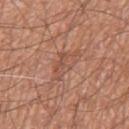<lesion>
  <biopsy_status>not biopsied; imaged during a skin examination</biopsy_status>
  <site>right thigh</site>
  <patient>
    <sex>male</sex>
    <age_approx>55</age_approx>
  </patient>
  <image>
    <source>total-body photography crop</source>
    <field_of_view_mm>15</field_of_view_mm>
  </image>
  <lighting>white-light</lighting>
  <lesion_size>
    <long_diameter_mm_approx>4.5</long_diameter_mm_approx>
  </lesion_size>
  <automated_metrics>
    <border_irregularity_0_10>4.5</border_irregularity_0_10>
    <nevus_likeness_0_100>0</nevus_likeness_0_100>
    <lesion_detection_confidence_0_100>95</lesion_detection_confidence_0_100>
  </automated_metrics>
</lesion>